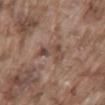Part of a total-body skin-imaging series; this lesion was reviewed on a skin check and was not flagged for biopsy.
A region of skin cropped from a whole-body photographic capture, roughly 15 mm wide.
A male patient approximately 75 years of age.
On the lower back.
The lesion's longest dimension is about 3.5 mm.
This is a white-light tile.